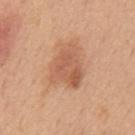On the mid back.
A male subject, roughly 50 years of age.
This is a white-light tile.
A lesion tile, about 15 mm wide, cut from a 3D total-body photograph.
The recorded lesion diameter is about 5 mm.